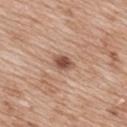No biopsy was performed on this lesion — it was imaged during a full skin examination and was not determined to be concerning. Measured at roughly 2.5 mm in maximum diameter. Imaged with white-light lighting. A roughly 15 mm field-of-view crop from a total-body skin photograph. Located on the upper back. A female subject, aged around 40.